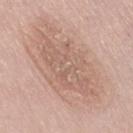<record>
  <biopsy_status>not biopsied; imaged during a skin examination</biopsy_status>
  <site>right thigh</site>
  <lesion_size>
    <long_diameter_mm_approx>9.0</long_diameter_mm_approx>
  </lesion_size>
  <image>
    <source>total-body photography crop</source>
    <field_of_view_mm>15</field_of_view_mm>
  </image>
  <lighting>white-light</lighting>
  <automated_metrics>
    <area_mm2_approx>23.0</area_mm2_approx>
    <eccentricity>0.9</eccentricity>
    <shape_asymmetry>0.25</shape_asymmetry>
    <peripheral_color_asymmetry>1.0</peripheral_color_asymmetry>
    <nevus_likeness_0_100>0</nevus_likeness_0_100>
  </automated_metrics>
  <patient>
    <sex>male</sex>
    <age_approx>55</age_approx>
  </patient>
</record>